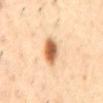| key | value |
|---|---|
| follow-up | total-body-photography surveillance lesion; no biopsy |
| lighting | cross-polarized |
| image source | ~15 mm crop, total-body skin-cancer survey |
| patient | male, in their mid-50s |
| location | the mid back |